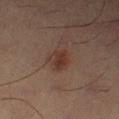The lesion was tiled from a total-body skin photograph and was not biopsied. Located on the right lower leg. Imaged with cross-polarized lighting. The patient is a male in their mid- to late 50s. This image is a 15 mm lesion crop taken from a total-body photograph. An algorithmic analysis of the crop reported a lesion color around L≈29 a*≈16 b*≈21 in CIELAB, roughly 6 lightness units darker than nearby skin, and a normalized border contrast of about 7.5. And it measured border irregularity of about 2.5 on a 0–10 scale, internal color variation of about 3.5 on a 0–10 scale, and peripheral color asymmetry of about 1.